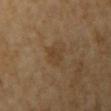Findings:
• follow-up · imaged on a skin check; not biopsied
• patient · female, aged around 70
• imaging modality · ~15 mm crop, total-body skin-cancer survey
• anatomic site · the arm